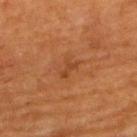Q: Was a biopsy performed?
A: imaged on a skin check; not biopsied
Q: What are the patient's age and sex?
A: male, aged around 60
Q: Automated lesion metrics?
A: an eccentricity of roughly 0.85; a border-irregularity index near 4.5/10
Q: Where on the body is the lesion?
A: the upper back
Q: What kind of image is this?
A: ~15 mm crop, total-body skin-cancer survey
Q: What lighting was used for the tile?
A: cross-polarized illumination